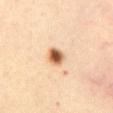Notes:
* notes · no biopsy performed (imaged during a skin exam)
* patient · female, in their mid-30s
* body site · the chest
* acquisition · ~15 mm crop, total-body skin-cancer survey
* tile lighting · cross-polarized